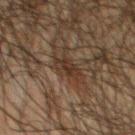Clinical impression:
Recorded during total-body skin imaging; not selected for excision or biopsy.
Context:
About 3 mm across. Located on the chest. A 15 mm close-up tile from a total-body photography series done for melanoma screening. A male subject, aged 58 to 62.